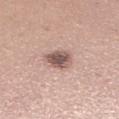No biopsy was performed on this lesion — it was imaged during a full skin examination and was not determined to be concerning.
The subject is a female in their 30s.
Measured at roughly 3 mm in maximum diameter.
On the right lower leg.
The tile uses white-light illumination.
This image is a 15 mm lesion crop taken from a total-body photograph.